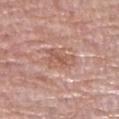follow-up: imaged on a skin check; not biopsied | tile lighting: white-light | location: the right lower leg | patient: female, aged 68 to 72 | image source: ~15 mm tile from a whole-body skin photo.